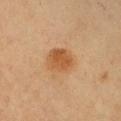<case>
  <biopsy_status>not biopsied; imaged during a skin examination</biopsy_status>
</case>